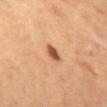Q: Was this lesion biopsied?
A: imaged on a skin check; not biopsied
Q: What are the patient's age and sex?
A: female
Q: How was the tile lit?
A: cross-polarized
Q: What is the anatomic site?
A: the abdomen
Q: What did automated image analysis measure?
A: a shape eccentricity near 0.8 and two-axis asymmetry of about 0.3; a lesion color around L≈55 a*≈25 b*≈37 in CIELAB and a lesion-to-skin contrast of about 10 (normalized; higher = more distinct); border irregularity of about 2.5 on a 0–10 scale, internal color variation of about 2.5 on a 0–10 scale, and radial color variation of about 0.5
Q: What kind of image is this?
A: ~15 mm tile from a whole-body skin photo
Q: How large is the lesion?
A: ~2.5 mm (longest diameter)